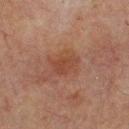notes = no biopsy performed (imaged during a skin exam) | image-analysis metrics = an average lesion color of about L≈35 a*≈19 b*≈25 (CIELAB), about 6 CIELAB-L* units darker than the surrounding skin, and a normalized lesion–skin contrast near 6; border irregularity of about 3 on a 0–10 scale, a color-variation rating of about 1.5/10, and peripheral color asymmetry of about 0.5; a nevus-likeness score of about 5/100 and a detector confidence of about 100 out of 100 that the crop contains a lesion | image source = total-body-photography crop, ~15 mm field of view | subject = male, about 65 years old | location = the upper back | lesion diameter = ≈4 mm.